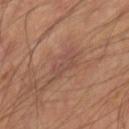workup — imaged on a skin check; not biopsied
diameter — ≈3.5 mm
lighting — cross-polarized
body site — the arm
subject — in their mid- to late 50s
image source — 15 mm crop, total-body photography
automated lesion analysis — a shape eccentricity near 0.8 and two-axis asymmetry of about 0.4; a lesion color around L≈47 a*≈20 b*≈25 in CIELAB and a lesion-to-skin contrast of about 5 (normalized; higher = more distinct); an automated nevus-likeness rating near 0 out of 100